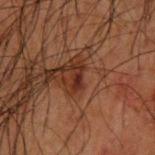No biopsy was performed on this lesion — it was imaged during a full skin examination and was not determined to be concerning. The recorded lesion diameter is about 3 mm. Located on the upper back. The patient is a male aged 48–52. Automated tile analysis of the lesion measured a lesion color around L≈21 a*≈19 b*≈22 in CIELAB, about 8 CIELAB-L* units darker than the surrounding skin, and a lesion-to-skin contrast of about 9.5 (normalized; higher = more distinct). The analysis additionally found a classifier nevus-likeness of about 5/100. A 15 mm crop from a total-body photograph taken for skin-cancer surveillance.